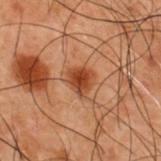biopsy_status: not biopsied; imaged during a skin examination
site: upper back
lesion_size:
  long_diameter_mm_approx: 3.0
patient:
  sex: male
  age_approx: 50
image:
  source: total-body photography crop
  field_of_view_mm: 15